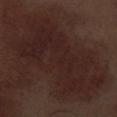Assessment: The lesion was tiled from a total-body skin photograph and was not biopsied. Image and clinical context: A male patient, aged around 70. The lesion is located on the left thigh. The total-body-photography lesion software estimated a border-irregularity rating of about 7/10, a within-lesion color-variation index near 2.5/10, and radial color variation of about 1. The tile uses white-light illumination. Longest diameter approximately 12 mm. A roughly 15 mm field-of-view crop from a total-body skin photograph.